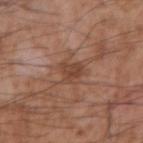<tbp_lesion>
  <biopsy_status>not biopsied; imaged during a skin examination</biopsy_status>
  <lighting>white-light</lighting>
  <patient>
    <sex>male</sex>
    <age_approx>75</age_approx>
  </patient>
  <lesion_size>
    <long_diameter_mm_approx>3.0</long_diameter_mm_approx>
  </lesion_size>
  <site>right upper arm</site>
  <automated_metrics>
    <area_mm2_approx>4.5</area_mm2_approx>
    <eccentricity>0.7</eccentricity>
    <shape_asymmetry>0.35</shape_asymmetry>
    <cielab_L>43</cielab_L>
    <cielab_a>21</cielab_a>
    <cielab_b>29</cielab_b>
    <vs_skin_contrast_norm>6.5</vs_skin_contrast_norm>
    <color_variation_0_10>2.0</color_variation_0_10>
    <peripheral_color_asymmetry>1.0</peripheral_color_asymmetry>
  </automated_metrics>
  <image>
    <source>total-body photography crop</source>
    <field_of_view_mm>15</field_of_view_mm>
  </image>
</tbp_lesion>